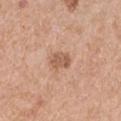Imaged during a routine full-body skin examination; the lesion was not biopsied and no histopathology is available. The recorded lesion diameter is about 2.5 mm. On the right upper arm. A region of skin cropped from a whole-body photographic capture, roughly 15 mm wide. Captured under white-light illumination. A male subject aged around 70.